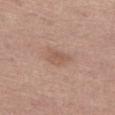Cropped from a whole-body photographic skin survey; the tile spans about 15 mm. Automated tile analysis of the lesion measured an average lesion color of about L≈55 a*≈19 b*≈28 (CIELAB), about 7 CIELAB-L* units darker than the surrounding skin, and a lesion-to-skin contrast of about 5.5 (normalized; higher = more distinct). And it measured border irregularity of about 3 on a 0–10 scale, internal color variation of about 2 on a 0–10 scale, and radial color variation of about 1. And it measured a detector confidence of about 100 out of 100 that the crop contains a lesion. A female subject, about 65 years old. Captured under white-light illumination. From the left thigh.